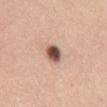Impression: The lesion was photographed on a routine skin check and not biopsied; there is no pathology result.